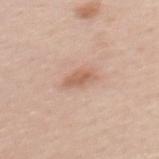No biopsy was performed on this lesion — it was imaged during a full skin examination and was not determined to be concerning.
About 3 mm across.
A female patient, aged 33–37.
From the upper back.
Captured under white-light illumination.
Cropped from a total-body skin-imaging series; the visible field is about 15 mm.
The lesion-visualizer software estimated a footprint of about 4.5 mm². It also reported border irregularity of about 2.5 on a 0–10 scale, internal color variation of about 1.5 on a 0–10 scale, and a peripheral color-asymmetry measure near 0.5. It also reported lesion-presence confidence of about 100/100.